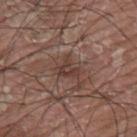Findings:
• notes: no biopsy performed (imaged during a skin exam)
• tile lighting: white-light
• image-analysis metrics: a color-variation rating of about 5/10 and peripheral color asymmetry of about 2; a nevus-likeness score of about 0/100 and lesion-presence confidence of about 50/100
• site: the upper back
• subject: male, aged 38 to 42
• diameter: about 3 mm
• imaging modality: total-body-photography crop, ~15 mm field of view